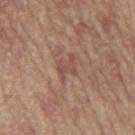Findings:
– workup: catalogued during a skin exam; not biopsied
– imaging modality: 15 mm crop, total-body photography
– body site: the mid back
– subject: male, aged around 65